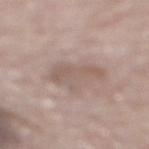The lesion was tiled from a total-body skin photograph and was not biopsied. This image is a 15 mm lesion crop taken from a total-body photograph. Captured under white-light illumination. A male subject, in their mid-80s or older. About 5 mm across. From the upper back.